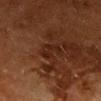Imaged during a routine full-body skin examination; the lesion was not biopsied and no histopathology is available.
A 15 mm close-up tile from a total-body photography series done for melanoma screening.
Longest diameter approximately 2.5 mm.
The tile uses cross-polarized illumination.
The patient is a female about 50 years old.
The lesion is located on the chest.